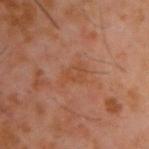Assessment: Part of a total-body skin-imaging series; this lesion was reviewed on a skin check and was not flagged for biopsy. Background: Measured at roughly 3.5 mm in maximum diameter. An algorithmic analysis of the crop reported a footprint of about 5.5 mm², a shape eccentricity near 0.75, and a shape-asymmetry score of about 0.3 (0 = symmetric). And it measured a nevus-likeness score of about 0/100 and a lesion-detection confidence of about 100/100. This image is a 15 mm lesion crop taken from a total-body photograph. A male patient, roughly 60 years of age. Located on the back.